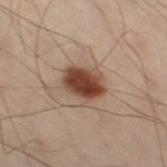Imaged during a routine full-body skin examination; the lesion was not biopsied and no histopathology is available.
A roughly 15 mm field-of-view crop from a total-body skin photograph.
On the left leg.
The patient is a male about 50 years old.
This is a cross-polarized tile.
Automated tile analysis of the lesion measured a lesion color around L≈35 a*≈16 b*≈23 in CIELAB and about 13 CIELAB-L* units darker than the surrounding skin. The analysis additionally found a nevus-likeness score of about 100/100 and a detector confidence of about 100 out of 100 that the crop contains a lesion.
Measured at roughly 4.5 mm in maximum diameter.